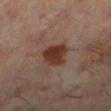{"biopsy_status": "not biopsied; imaged during a skin examination", "automated_metrics": {"cielab_L": 36, "cielab_a": 20, "cielab_b": 27, "vs_skin_darker_L": 12.0, "vs_skin_contrast_norm": 10.5, "nevus_likeness_0_100": 95, "lesion_detection_confidence_0_100": 100}, "lesion_size": {"long_diameter_mm_approx": 3.5}, "lighting": "cross-polarized", "image": {"source": "total-body photography crop", "field_of_view_mm": 15}, "site": "left lower leg", "patient": {"sex": "female", "age_approx": 60}}